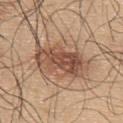Recorded during total-body skin imaging; not selected for excision or biopsy.